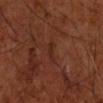The lesion was photographed on a routine skin check and not biopsied; there is no pathology result. Cropped from a whole-body photographic skin survey; the tile spans about 15 mm. The lesion is on the right forearm. A male subject aged 58 to 62.